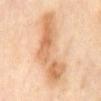• automated metrics — an average lesion color of about L≈67 a*≈20 b*≈37 (CIELAB) and a normalized lesion–skin contrast near 7.5; a border-irregularity rating of about 5.5/10 and a peripheral color-asymmetry measure near 2; a lesion-detection confidence of about 100/100
• lighting — cross-polarized
• subject — female, aged approximately 50
• site — the abdomen
• image — 15 mm crop, total-body photography
• lesion diameter — ~10 mm (longest diameter)